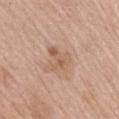No biopsy was performed on this lesion — it was imaged during a full skin examination and was not determined to be concerning. A male subject roughly 60 years of age. A close-up tile cropped from a whole-body skin photograph, about 15 mm across. From the right upper arm.